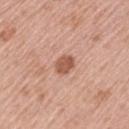{
  "biopsy_status": "not biopsied; imaged during a skin examination",
  "image": {
    "source": "total-body photography crop",
    "field_of_view_mm": 15
  },
  "patient": {
    "sex": "male",
    "age_approx": 40
  },
  "site": "left upper arm",
  "lighting": "white-light"
}